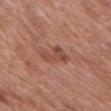The recorded lesion diameter is about 3.5 mm.
On the left upper arm.
A 15 mm close-up extracted from a 3D total-body photography capture.
A male subject about 55 years old.